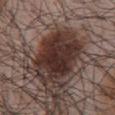The lesion was tiled from a total-body skin photograph and was not biopsied. This is a white-light tile. A male patient in their mid- to late 60s. Cropped from a whole-body photographic skin survey; the tile spans about 15 mm. Approximately 7 mm at its widest. Located on the front of the torso. The total-body-photography lesion software estimated a shape eccentricity near 0.65 and a shape-asymmetry score of about 0.15 (0 = symmetric). The analysis additionally found a nevus-likeness score of about 100/100 and a detector confidence of about 100 out of 100 that the crop contains a lesion.